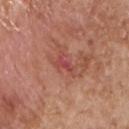{
  "biopsy_status": "not biopsied; imaged during a skin examination",
  "patient": {
    "sex": "male",
    "age_approx": 65
  },
  "lighting": "white-light",
  "site": "chest",
  "lesion_size": {
    "long_diameter_mm_approx": 2.5
  },
  "image": {
    "source": "total-body photography crop",
    "field_of_view_mm": 15
  },
  "automated_metrics": {
    "cielab_L": 47,
    "cielab_a": 32,
    "cielab_b": 26,
    "vs_skin_darker_L": 8.0,
    "vs_skin_contrast_norm": 6.0,
    "border_irregularity_0_10": 4.0,
    "color_variation_0_10": 0.0,
    "peripheral_color_asymmetry": 0.0,
    "nevus_likeness_0_100": 0,
    "lesion_detection_confidence_0_100": 90
  }
}